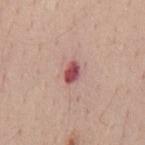{
  "image": {
    "source": "total-body photography crop",
    "field_of_view_mm": 15
  },
  "lesion_size": {
    "long_diameter_mm_approx": 2.5
  },
  "site": "mid back",
  "patient": {
    "sex": "male",
    "age_approx": 40
  },
  "lighting": "white-light"
}